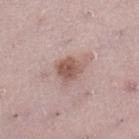Part of a total-body skin-imaging series; this lesion was reviewed on a skin check and was not flagged for biopsy.
Measured at roughly 3.5 mm in maximum diameter.
A region of skin cropped from a whole-body photographic capture, roughly 15 mm wide.
The subject is a female roughly 25 years of age.
The lesion is located on the right lower leg.
The tile uses white-light illumination.
Automated image analysis of the tile measured a footprint of about 7.5 mm², a shape eccentricity near 0.6, and a symmetry-axis asymmetry near 0.25. The software also gave a lesion color around L≈56 a*≈19 b*≈24 in CIELAB, a lesion–skin lightness drop of about 11, and a normalized border contrast of about 8. The software also gave a border-irregularity rating of about 2.5/10, internal color variation of about 4 on a 0–10 scale, and peripheral color asymmetry of about 1.5.